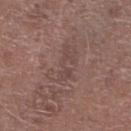This lesion was catalogued during total-body skin photography and was not selected for biopsy.
The lesion's longest dimension is about 5 mm.
Located on the leg.
A roughly 15 mm field-of-view crop from a total-body skin photograph.
A male patient, aged approximately 75.